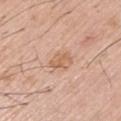<case>
  <biopsy_status>not biopsied; imaged during a skin examination</biopsy_status>
  <image>
    <source>total-body photography crop</source>
    <field_of_view_mm>15</field_of_view_mm>
  </image>
  <lighting>white-light</lighting>
  <lesion_size>
    <long_diameter_mm_approx>3.0</long_diameter_mm_approx>
  </lesion_size>
  <patient>
    <sex>male</sex>
    <age_approx>55</age_approx>
  </patient>
</case>